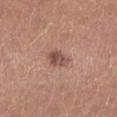• follow-up: imaged on a skin check; not biopsied
• patient: female, in their 40s
• location: the left lower leg
• image-analysis metrics: an area of roughly 4.5 mm², a shape eccentricity near 0.75, and two-axis asymmetry of about 0.25; an average lesion color of about L≈50 a*≈21 b*≈25 (CIELAB) and a lesion-to-skin contrast of about 8 (normalized; higher = more distinct); a border-irregularity index near 2.5/10, a color-variation rating of about 5/10, and peripheral color asymmetry of about 2
• image source: 15 mm crop, total-body photography
• diameter: ~3 mm (longest diameter)